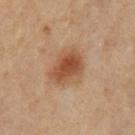The lesion was tiled from a total-body skin photograph and was not biopsied. A lesion tile, about 15 mm wide, cut from a 3D total-body photograph. The lesion is on the right thigh. A female patient aged 48 to 52.